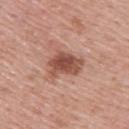Impression:
The lesion was photographed on a routine skin check and not biopsied; there is no pathology result.
Image and clinical context:
Located on the back. A male patient aged 53–57. Cropped from a total-body skin-imaging series; the visible field is about 15 mm.